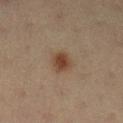The lesion was tiled from a total-body skin photograph and was not biopsied.
The lesion is on the leg.
A female subject, aged around 20.
A 15 mm close-up tile from a total-body photography series done for melanoma screening.
The recorded lesion diameter is about 2.5 mm.
Captured under cross-polarized illumination.